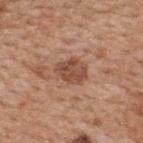<lesion>
<site>back</site>
<image>
  <source>total-body photography crop</source>
  <field_of_view_mm>15</field_of_view_mm>
</image>
<patient>
  <sex>male</sex>
  <age_approx>65</age_approx>
</patient>
</lesion>